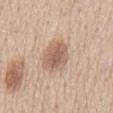<record>
  <biopsy_status>not biopsied; imaged during a skin examination</biopsy_status>
  <lighting>white-light</lighting>
  <automated_metrics>
    <area_mm2_approx>11.0</area_mm2_approx>
    <eccentricity>0.8</eccentricity>
    <shape_asymmetry>0.15</shape_asymmetry>
  </automated_metrics>
  <image>
    <source>total-body photography crop</source>
    <field_of_view_mm>15</field_of_view_mm>
  </image>
  <patient>
    <sex>male</sex>
    <age_approx>60</age_approx>
  </patient>
  <site>front of the torso</site>
</record>